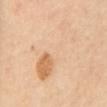Case summary:
• follow-up — catalogued during a skin exam; not biopsied
• imaging modality — total-body-photography crop, ~15 mm field of view
• location — the mid back
• automated metrics — an area of roughly 26 mm², a shape eccentricity near 0.9, and a shape-asymmetry score of about 0.55 (0 = symmetric)
• patient — female, about 55 years old
• lighting — cross-polarized illumination